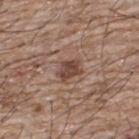size: ≈3 mm
image source: ~15 mm tile from a whole-body skin photo
anatomic site: the upper back
subject: male, roughly 65 years of age